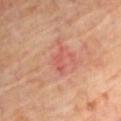The lesion was photographed on a routine skin check and not biopsied; there is no pathology result. The tile uses cross-polarized illumination. Automated image analysis of the tile measured a footprint of about 3.5 mm². The software also gave a border-irregularity rating of about 5.5/10, internal color variation of about 1.5 on a 0–10 scale, and peripheral color asymmetry of about 0. And it measured a detector confidence of about 100 out of 100 that the crop contains a lesion. The recorded lesion diameter is about 3.5 mm. A male patient, aged approximately 60. Located on the front of the torso. A 15 mm close-up extracted from a 3D total-body photography capture.